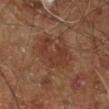The recorded lesion diameter is about 5 mm.
The subject is a male aged around 60.
A region of skin cropped from a whole-body photographic capture, roughly 15 mm wide.
Imaged with cross-polarized lighting.
The lesion-visualizer software estimated an eccentricity of roughly 0.75 and two-axis asymmetry of about 0.4. The software also gave a classifier nevus-likeness of about 0/100 and lesion-presence confidence of about 100/100.
On the leg.